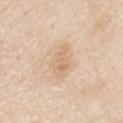| feature | finding |
|---|---|
| lesion size | ~4 mm (longest diameter) |
| image | 15 mm crop, total-body photography |
| patient | male, aged around 80 |
| automated metrics | a footprint of about 8 mm², a shape eccentricity near 0.8, and a shape-asymmetry score of about 0.2 (0 = symmetric); an average lesion color of about L≈70 a*≈16 b*≈33 (CIELAB), roughly 7 lightness units darker than nearby skin, and a lesion-to-skin contrast of about 5 (normalized; higher = more distinct); a color-variation rating of about 2.5/10 and peripheral color asymmetry of about 1; an automated nevus-likeness rating near 0 out of 100 and a lesion-detection confidence of about 100/100 |
| body site | the right upper arm |
| lighting | white-light illumination |